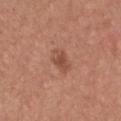{
  "patient": {
    "sex": "female",
    "age_approx": 40
  },
  "lighting": "white-light",
  "site": "chest",
  "image": {
    "source": "total-body photography crop",
    "field_of_view_mm": 15
  }
}